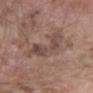workup: imaged on a skin check; not biopsied | anatomic site: the left forearm | patient: male, roughly 60 years of age | lighting: white-light | imaging modality: 15 mm crop, total-body photography.